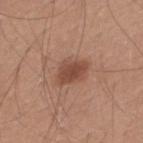workup: no biopsy performed (imaged during a skin exam) | image source: ~15 mm crop, total-body skin-cancer survey | size: ~3.5 mm (longest diameter) | automated lesion analysis: an area of roughly 7 mm², an eccentricity of roughly 0.75, and two-axis asymmetry of about 0.3; a border-irregularity index near 3/10, a color-variation rating of about 2.5/10, and peripheral color asymmetry of about 0.5; an automated nevus-likeness rating near 90 out of 100 and a lesion-detection confidence of about 100/100 | subject: male, roughly 30 years of age | anatomic site: the upper back.